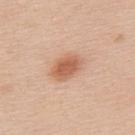This lesion was catalogued during total-body skin photography and was not selected for biopsy. The lesion is located on the upper back. Imaged with white-light lighting. A roughly 15 mm field-of-view crop from a total-body skin photograph. The patient is a female aged 43–47. Automated image analysis of the tile measured a footprint of about 9 mm² and two-axis asymmetry of about 0.2.